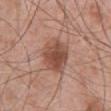anatomic site: the abdomen
diameter: ≈4 mm
subject: male, aged 68–72
acquisition: 15 mm crop, total-body photography